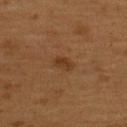Clinical impression:
The lesion was photographed on a routine skin check and not biopsied; there is no pathology result.
Clinical summary:
The tile uses cross-polarized illumination. A 15 mm crop from a total-body photograph taken for skin-cancer surveillance. Measured at roughly 2.5 mm in maximum diameter. Located on the upper back. A male patient aged around 55.